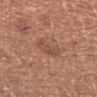Impression: Imaged during a routine full-body skin examination; the lesion was not biopsied and no histopathology is available. Context: The recorded lesion diameter is about 3 mm. A roughly 15 mm field-of-view crop from a total-body skin photograph. A female subject, aged approximately 35. This is a white-light tile. On the left upper arm. The total-body-photography lesion software estimated a footprint of about 5 mm², a shape eccentricity near 0.75, and a symmetry-axis asymmetry near 0.35. It also reported a mean CIELAB color near L≈49 a*≈22 b*≈26 and a normalized border contrast of about 5.5.